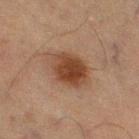Captured under cross-polarized illumination.
A close-up tile cropped from a whole-body skin photograph, about 15 mm across.
A male subject, in their mid-60s.
Located on the left thigh.
Approximately 4 mm at its widest.